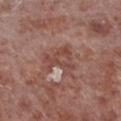Findings:
* lesion size: about 4 mm
* body site: the leg
* acquisition: 15 mm crop, total-body photography
* patient: male, in their mid- to late 50s
* automated lesion analysis: an eccentricity of roughly 0.7 and a symmetry-axis asymmetry near 0.45